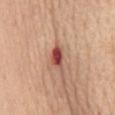workup=catalogued during a skin exam; not biopsied
image source=~15 mm tile from a whole-body skin photo
patient=female, roughly 65 years of age
body site=the mid back
lesion size=about 3 mm